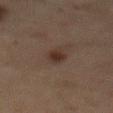The lesion was photographed on a routine skin check and not biopsied; there is no pathology result.
Longest diameter approximately 3.5 mm.
This image is a 15 mm lesion crop taken from a total-body photograph.
Captured under cross-polarized illumination.
A male patient in their mid-70s.
From the abdomen.